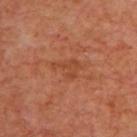| field | value |
|---|---|
| follow-up | no biopsy performed (imaged during a skin exam) |
| image | 15 mm crop, total-body photography |
| subject | male, aged 68–72 |
| tile lighting | cross-polarized |
| body site | the upper back |
| diameter | about 3.5 mm |
| image-analysis metrics | about 6 CIELAB-L* units darker than the surrounding skin and a normalized border contrast of about 5 |